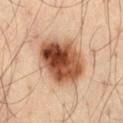Captured during whole-body skin photography for melanoma surveillance; the lesion was not biopsied.
The recorded lesion diameter is about 6 mm.
A roughly 15 mm field-of-view crop from a total-body skin photograph.
A male subject, in their mid- to late 30s.
The lesion is on the left thigh.